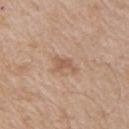Q: Was this lesion biopsied?
A: catalogued during a skin exam; not biopsied
Q: How was the tile lit?
A: white-light
Q: How was this image acquired?
A: total-body-photography crop, ~15 mm field of view
Q: Patient demographics?
A: male, approximately 65 years of age
Q: What is the anatomic site?
A: the right upper arm